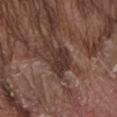Assessment:
This lesion was catalogued during total-body skin photography and was not selected for biopsy.
Context:
From the abdomen. A male patient, aged around 80. Cropped from a whole-body photographic skin survey; the tile spans about 15 mm.